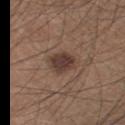Notes:
• follow-up · total-body-photography surveillance lesion; no biopsy
• subject · male, aged approximately 45
• tile lighting · white-light
• lesion diameter · ~3.5 mm (longest diameter)
• anatomic site · the left thigh
• image-analysis metrics · a classifier nevus-likeness of about 85/100 and lesion-presence confidence of about 100/100
• acquisition · ~15 mm tile from a whole-body skin photo